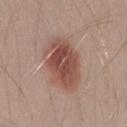follow-up: no biopsy performed (imaged during a skin exam); acquisition: 15 mm crop, total-body photography; body site: the leg; diameter: ~6.5 mm (longest diameter); patient: male, about 30 years old; tile lighting: white-light.